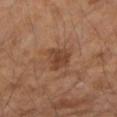Case summary:
- follow-up: catalogued during a skin exam; not biopsied
- location: the right forearm
- imaging modality: ~15 mm crop, total-body skin-cancer survey
- size: ~3.5 mm (longest diameter)
- lighting: cross-polarized illumination
- subject: female, in their 60s
- image-analysis metrics: a lesion area of about 7 mm² and a symmetry-axis asymmetry near 0.25; a lesion–skin lightness drop of about 9 and a normalized lesion–skin contrast near 7; a color-variation rating of about 2.5/10 and radial color variation of about 1